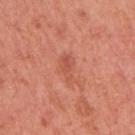Q: How was this image acquired?
A: total-body-photography crop, ~15 mm field of view
Q: Where on the body is the lesion?
A: the right upper arm
Q: What did automated image analysis measure?
A: a lesion color around L≈55 a*≈31 b*≈33 in CIELAB and about 7 CIELAB-L* units darker than the surrounding skin; a nevus-likeness score of about 0/100
Q: What lighting was used for the tile?
A: white-light
Q: Who is the patient?
A: female, aged 48 to 52